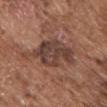follow-up — catalogued during a skin exam; not biopsied
image source — ~15 mm tile from a whole-body skin photo
size — ~6.5 mm (longest diameter)
subject — male, roughly 75 years of age
TBP lesion metrics — a footprint of about 17 mm², an outline eccentricity of about 0.8 (0 = round, 1 = elongated), and two-axis asymmetry of about 0.25; an automated nevus-likeness rating near 5 out of 100 and a detector confidence of about 100 out of 100 that the crop contains a lesion
anatomic site — the chest